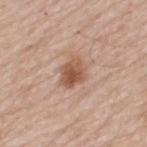No biopsy was performed on this lesion — it was imaged during a full skin examination and was not determined to be concerning. Automated image analysis of the tile measured about 12 CIELAB-L* units darker than the surrounding skin and a lesion-to-skin contrast of about 8.5 (normalized; higher = more distinct). A 15 mm close-up tile from a total-body photography series done for melanoma screening. The patient is a male in their mid-60s. The recorded lesion diameter is about 3.5 mm. On the back. The tile uses white-light illumination.